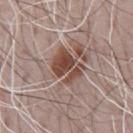workup: no biopsy performed (imaged during a skin exam); imaging modality: total-body-photography crop, ~15 mm field of view; patient: male, aged around 70; anatomic site: the chest; tile lighting: white-light; diameter: about 4 mm.